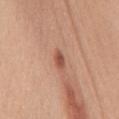Impression: The lesion was tiled from a total-body skin photograph and was not biopsied. Acquisition and patient details: A female patient, in their mid- to late 40s. Located on the chest. Cropped from a whole-body photographic skin survey; the tile spans about 15 mm. The total-body-photography lesion software estimated a footprint of about 3 mm², an eccentricity of roughly 0.85, and a shape-asymmetry score of about 0.3 (0 = symmetric). The software also gave border irregularity of about 3 on a 0–10 scale, internal color variation of about 2 on a 0–10 scale, and peripheral color asymmetry of about 0.5.